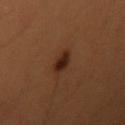Captured during whole-body skin photography for melanoma surveillance; the lesion was not biopsied. Longest diameter approximately 2.5 mm. A female patient aged 38 to 42. A 15 mm crop from a total-body photograph taken for skin-cancer surveillance. Imaged with cross-polarized lighting. From the right upper arm.